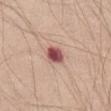biopsy status: imaged on a skin check; not biopsied | automated lesion analysis: a lesion area of about 5 mm², an outline eccentricity of about 0.6 (0 = round, 1 = elongated), and a symmetry-axis asymmetry near 0.25 | lighting: white-light | image: 15 mm crop, total-body photography | body site: the left thigh | diameter: ≈2.5 mm | patient: male, aged approximately 65.